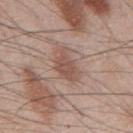{
  "image": {
    "source": "total-body photography crop",
    "field_of_view_mm": 15
  },
  "site": "back",
  "patient": {
    "sex": "male",
    "age_approx": 45
  }
}